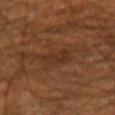Imaged during a routine full-body skin examination; the lesion was not biopsied and no histopathology is available. The lesion is located on the right forearm. A 15 mm close-up extracted from a 3D total-body photography capture. A male patient aged 48 to 52. Longest diameter approximately 3.5 mm. Captured under cross-polarized illumination.